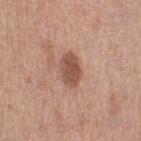The lesion was photographed on a routine skin check and not biopsied; there is no pathology result.
The recorded lesion diameter is about 4 mm.
Located on the left thigh.
The total-body-photography lesion software estimated an eccentricity of roughly 0.8 and a symmetry-axis asymmetry near 0.2. The analysis additionally found a mean CIELAB color near L≈52 a*≈22 b*≈27, about 12 CIELAB-L* units darker than the surrounding skin, and a lesion-to-skin contrast of about 8.5 (normalized; higher = more distinct). It also reported an automated nevus-likeness rating near 65 out of 100 and lesion-presence confidence of about 100/100.
A roughly 15 mm field-of-view crop from a total-body skin photograph.
A female patient aged 38–42.
Captured under white-light illumination.